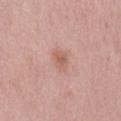Clinical impression:
The lesion was tiled from a total-body skin photograph and was not biopsied.
Context:
From the abdomen. A female subject, aged 38 to 42. A 15 mm crop from a total-body photograph taken for skin-cancer surveillance. Automated image analysis of the tile measured a classifier nevus-likeness of about 0/100 and a detector confidence of about 100 out of 100 that the crop contains a lesion. Longest diameter approximately 2.5 mm. This is a white-light tile.